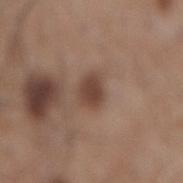Captured under white-light illumination. A roughly 15 mm field-of-view crop from a total-body skin photograph. The lesion's longest dimension is about 3 mm. The total-body-photography lesion software estimated border irregularity of about 2 on a 0–10 scale, a within-lesion color-variation index near 2/10, and a peripheral color-asymmetry measure near 0.5. It also reported a nevus-likeness score of about 85/100 and a detector confidence of about 100 out of 100 that the crop contains a lesion. The lesion is located on the mid back. A male patient, aged 58–62.